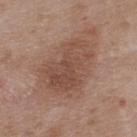Imaged during a routine full-body skin examination; the lesion was not biopsied and no histopathology is available. The lesion-visualizer software estimated an average lesion color of about L≈48 a*≈19 b*≈26 (CIELAB) and about 8 CIELAB-L* units darker than the surrounding skin. And it measured a classifier nevus-likeness of about 15/100 and a detector confidence of about 100 out of 100 that the crop contains a lesion. Imaged with white-light lighting. A lesion tile, about 15 mm wide, cut from a 3D total-body photograph. The subject is a male about 50 years old. From the upper back.